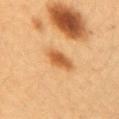Q: Was this lesion biopsied?
A: no biopsy performed (imaged during a skin exam)
Q: How was this image acquired?
A: ~15 mm tile from a whole-body skin photo
Q: What is the lesion's diameter?
A: ≈3 mm
Q: Where on the body is the lesion?
A: the arm
Q: Who is the patient?
A: female, about 40 years old
Q: What did automated image analysis measure?
A: a lesion color around L≈51 a*≈22 b*≈39 in CIELAB, a lesion–skin lightness drop of about 11, and a lesion-to-skin contrast of about 8.5 (normalized; higher = more distinct); a within-lesion color-variation index near 2/10 and radial color variation of about 0.5